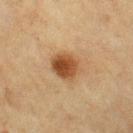| feature | finding |
|---|---|
| notes | total-body-photography surveillance lesion; no biopsy |